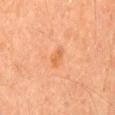– notes · catalogued during a skin exam; not biopsied
– subject · male, in their mid- to late 60s
– image · ~15 mm crop, total-body skin-cancer survey
– tile lighting · cross-polarized illumination
– lesion size · about 3 mm
– anatomic site · the mid back